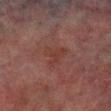Part of a total-body skin-imaging series; this lesion was reviewed on a skin check and was not flagged for biopsy.
Measured at roughly 3 mm in maximum diameter.
This image is a 15 mm lesion crop taken from a total-body photograph.
A male patient approximately 75 years of age.
Located on the right lower leg.